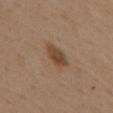| key | value |
|---|---|
| biopsy status | no biopsy performed (imaged during a skin exam) |
| subject | female, aged 38–42 |
| imaging modality | 15 mm crop, total-body photography |
| lesion size | about 3.5 mm |
| site | the upper back |
| illumination | white-light illumination |